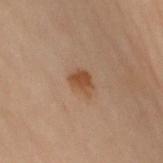Assessment:
This lesion was catalogued during total-body skin photography and was not selected for biopsy.
Clinical summary:
Imaged with cross-polarized lighting. Automated image analysis of the tile measured an area of roughly 4.5 mm², an outline eccentricity of about 0.6 (0 = round, 1 = elongated), and two-axis asymmetry of about 0.2. And it measured a lesion color around L≈41 a*≈18 b*≈30 in CIELAB and roughly 8 lightness units darker than nearby skin. The analysis additionally found border irregularity of about 2 on a 0–10 scale, internal color variation of about 2.5 on a 0–10 scale, and a peripheral color-asymmetry measure near 1. The analysis additionally found an automated nevus-likeness rating near 90 out of 100 and lesion-presence confidence of about 100/100. Located on the left arm. A female subject aged 58–62. A 15 mm close-up tile from a total-body photography series done for melanoma screening. The recorded lesion diameter is about 2.5 mm.